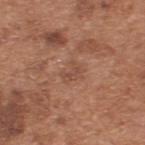biopsy_status: not biopsied; imaged during a skin examination
patient:
  sex: male
  age_approx: 65
automated_metrics:
  eccentricity: 0.7
  shape_asymmetry: 0.4
  cielab_L: 49
  cielab_a: 22
  cielab_b: 29
  vs_skin_darker_L: 6.0
  vs_skin_contrast_norm: 5.0
  color_variation_0_10: 2.0
  peripheral_color_asymmetry: 0.5
  nevus_likeness_0_100: 0
  lesion_detection_confidence_0_100: 100
image:
  source: total-body photography crop
  field_of_view_mm: 15
site: back